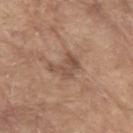| key | value |
|---|---|
| lighting | white-light |
| acquisition | 15 mm crop, total-body photography |
| site | the left upper arm |
| subject | male, approximately 80 years of age |
| diameter | about 3.5 mm |